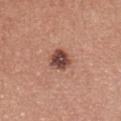This lesion was catalogued during total-body skin photography and was not selected for biopsy.
The lesion is on the chest.
The subject is a female in their mid- to late 20s.
Cropped from a total-body skin-imaging series; the visible field is about 15 mm.